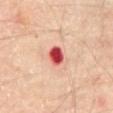Impression:
The lesion was tiled from a total-body skin photograph and was not biopsied.
Background:
Captured under cross-polarized illumination. The patient is a male in their mid- to late 60s. A 15 mm crop from a total-body photograph taken for skin-cancer surveillance. An algorithmic analysis of the crop reported a footprint of about 5 mm² and an eccentricity of roughly 0.6. And it measured a mean CIELAB color near L≈52 a*≈42 b*≈30 and a lesion-to-skin contrast of about 13 (normalized; higher = more distinct). The software also gave an automated nevus-likeness rating near 0 out of 100 and a lesion-detection confidence of about 100/100. On the abdomen.